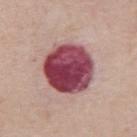lesion size=about 6 mm | body site=the abdomen | patient=male, aged 68–72 | image-analysis metrics=an area of roughly 28 mm² and two-axis asymmetry of about 0.1; an automated nevus-likeness rating near 95 out of 100 and a detector confidence of about 95 out of 100 that the crop contains a lesion | acquisition=total-body-photography crop, ~15 mm field of view.